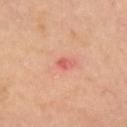notes = catalogued during a skin exam; not biopsied | diameter = about 1.5 mm | subject = female, roughly 65 years of age | location = the right upper arm | image-analysis metrics = an area of roughly 1.5 mm², an eccentricity of roughly 0.4, and a symmetry-axis asymmetry near 0.4; a lesion color around L≈61 a*≈35 b*≈29 in CIELAB, about 10 CIELAB-L* units darker than the surrounding skin, and a normalized border contrast of about 6.5; a border-irregularity index near 3/10, internal color variation of about 1 on a 0–10 scale, and radial color variation of about 0; a nevus-likeness score of about 0/100 and a detector confidence of about 100 out of 100 that the crop contains a lesion | imaging modality = ~15 mm crop, total-body skin-cancer survey | lighting = cross-polarized.